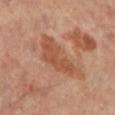Clinical impression:
No biopsy was performed on this lesion — it was imaged during a full skin examination and was not determined to be concerning.
Clinical summary:
The tile uses cross-polarized illumination. Located on the left lower leg. A female patient, about 60 years old. A 15 mm crop from a total-body photograph taken for skin-cancer surveillance. An algorithmic analysis of the crop reported a footprint of about 13 mm², an eccentricity of roughly 0.9, and a symmetry-axis asymmetry near 0.45. It also reported a lesion–skin lightness drop of about 9 and a normalized border contrast of about 6.5. And it measured a peripheral color-asymmetry measure near 1. The analysis additionally found a nevus-likeness score of about 0/100 and a detector confidence of about 100 out of 100 that the crop contains a lesion. Approximately 6.5 mm at its widest.